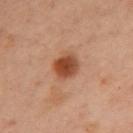The lesion was tiled from a total-body skin photograph and was not biopsied.
An algorithmic analysis of the crop reported a footprint of about 7.5 mm² and an outline eccentricity of about 0.65 (0 = round, 1 = elongated). The software also gave a detector confidence of about 100 out of 100 that the crop contains a lesion.
The lesion's longest dimension is about 3.5 mm.
The lesion is located on the front of the torso.
A 15 mm close-up extracted from a 3D total-body photography capture.
The patient is a female approximately 55 years of age.
Imaged with cross-polarized lighting.